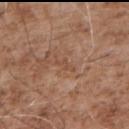{"biopsy_status": "not biopsied; imaged during a skin examination", "image": {"source": "total-body photography crop", "field_of_view_mm": 15}, "site": "upper back", "patient": {"sex": "male", "age_approx": 55}, "lesion_size": {"long_diameter_mm_approx": 2.5}}